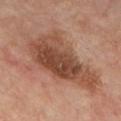Clinical impression:
Imaged during a routine full-body skin examination; the lesion was not biopsied and no histopathology is available.
Clinical summary:
On the chest. Cropped from a whole-body photographic skin survey; the tile spans about 15 mm. The subject is about 55 years old.